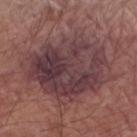Clinical impression: The lesion was photographed on a routine skin check and not biopsied; there is no pathology result. Acquisition and patient details: On the right forearm. The lesion's longest dimension is about 10 mm. Automated image analysis of the tile measured border irregularity of about 3.5 on a 0–10 scale, a color-variation rating of about 7.5/10, and a peripheral color-asymmetry measure near 2.5. A 15 mm close-up tile from a total-body photography series done for melanoma screening. The tile uses white-light illumination. A male patient, roughly 65 years of age.